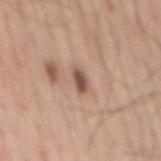biopsy status = no biopsy performed (imaged during a skin exam) | location = the mid back | image = total-body-photography crop, ~15 mm field of view | patient = male, aged approximately 60 | lesion size = about 2.5 mm | automated lesion analysis = a mean CIELAB color near L≈51 a*≈19 b*≈25 and a normalized border contrast of about 9.5; border irregularity of about 3 on a 0–10 scale and internal color variation of about 2 on a 0–10 scale | lighting = white-light illumination.